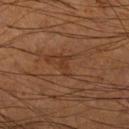Imaged during a routine full-body skin examination; the lesion was not biopsied and no histopathology is available.
The lesion-visualizer software estimated roughly 5 lightness units darker than nearby skin. The analysis additionally found a color-variation rating of about 0/10 and a peripheral color-asymmetry measure near 0.
On the right lower leg.
Imaged with cross-polarized lighting.
A male patient aged approximately 55.
A 15 mm crop from a total-body photograph taken for skin-cancer surveillance.
About 3 mm across.